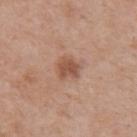Captured during whole-body skin photography for melanoma surveillance; the lesion was not biopsied. Measured at roughly 2.5 mm in maximum diameter. The tile uses white-light illumination. From the front of the torso. A 15 mm crop from a total-body photograph taken for skin-cancer surveillance. A male patient aged approximately 70.